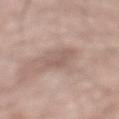Captured during whole-body skin photography for melanoma surveillance; the lesion was not biopsied. Captured under white-light illumination. Located on the mid back. An algorithmic analysis of the crop reported a shape eccentricity near 0.85 and two-axis asymmetry of about 0.6. It also reported a border-irregularity rating of about 6.5/10 and a peripheral color-asymmetry measure near 0. It also reported a detector confidence of about 90 out of 100 that the crop contains a lesion. Cropped from a total-body skin-imaging series; the visible field is about 15 mm. The subject is a male about 60 years old. Longest diameter approximately 2.5 mm.